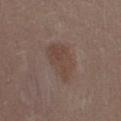This lesion was catalogued during total-body skin photography and was not selected for biopsy.
Cropped from a whole-body photographic skin survey; the tile spans about 15 mm.
A female subject, in their 30s.
The lesion is on the leg.